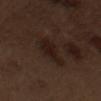Impression: This lesion was catalogued during total-body skin photography and was not selected for biopsy. Clinical summary: The lesion's longest dimension is about 4 mm. A region of skin cropped from a whole-body photographic capture, roughly 15 mm wide. On the left upper arm. An algorithmic analysis of the crop reported an area of roughly 10 mm², a shape eccentricity near 0.7, and a shape-asymmetry score of about 0.3 (0 = symmetric). The analysis additionally found an average lesion color of about L≈19 a*≈14 b*≈18 (CIELAB), roughly 6 lightness units darker than nearby skin, and a lesion-to-skin contrast of about 8 (normalized; higher = more distinct). The analysis additionally found a border-irregularity index near 3.5/10 and radial color variation of about 1. The analysis additionally found a detector confidence of about 100 out of 100 that the crop contains a lesion. The tile uses white-light illumination. A male patient aged around 70.